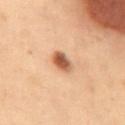A roughly 15 mm field-of-view crop from a total-body skin photograph.
About 3 mm across.
The subject is a male aged around 55.
The lesion is located on the abdomen.
The lesion-visualizer software estimated an area of roughly 5.5 mm² and a shape eccentricity near 0.65. The software also gave a mean CIELAB color near L≈47 a*≈19 b*≈30, roughly 13 lightness units darker than nearby skin, and a normalized border contrast of about 9.5. The software also gave a color-variation rating of about 5.5/10 and a peripheral color-asymmetry measure near 1.5.